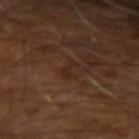This lesion was catalogued during total-body skin photography and was not selected for biopsy. The patient is a male in their 60s. A roughly 15 mm field-of-view crop from a total-body skin photograph. The tile uses cross-polarized illumination. About 3 mm across. The lesion is located on the arm.